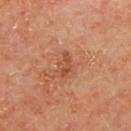<lesion>
<biopsy_status>not biopsied; imaged during a skin examination</biopsy_status>
<lighting>cross-polarized</lighting>
<patient>
  <sex>male</sex>
  <age_approx>65</age_approx>
</patient>
<site>mid back</site>
<lesion_size>
  <long_diameter_mm_approx>3.0</long_diameter_mm_approx>
</lesion_size>
<automated_metrics>
  <area_mm2_approx>3.5</area_mm2_approx>
  <eccentricity>0.85</eccentricity>
  <shape_asymmetry>0.3</shape_asymmetry>
  <border_irregularity_0_10>3.5</border_irregularity_0_10>
  <color_variation_0_10>1.5</color_variation_0_10>
  <peripheral_color_asymmetry>0.5</peripheral_color_asymmetry>
  <nevus_likeness_0_100>0</nevus_likeness_0_100>
  <lesion_detection_confidence_0_100>100</lesion_detection_confidence_0_100>
</automated_metrics>
<image>
  <source>total-body photography crop</source>
  <field_of_view_mm>15</field_of_view_mm>
</image>
</lesion>